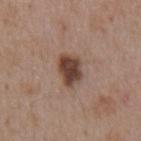No biopsy was performed on this lesion — it was imaged during a full skin examination and was not determined to be concerning. A 15 mm close-up tile from a total-body photography series done for melanoma screening. The lesion is on the abdomen. This is a white-light tile. A male patient, in their mid- to late 50s.